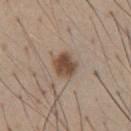Findings:
* workup · imaged on a skin check; not biopsied
* location · the chest
* lesion diameter · about 3 mm
* illumination · white-light
* image · ~15 mm tile from a whole-body skin photo
* subject · male, approximately 55 years of age